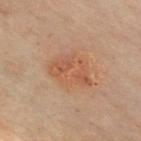Captured during whole-body skin photography for melanoma surveillance; the lesion was not biopsied.
A close-up tile cropped from a whole-body skin photograph, about 15 mm across.
This is a cross-polarized tile.
A male patient, aged 48–52.
From the chest.